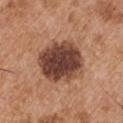Recorded during total-body skin imaging; not selected for excision or biopsy.
Longest diameter approximately 6 mm.
The lesion-visualizer software estimated a footprint of about 23 mm², a shape eccentricity near 0.55, and a symmetry-axis asymmetry near 0.1. It also reported an average lesion color of about L≈42 a*≈22 b*≈27 (CIELAB), about 18 CIELAB-L* units darker than the surrounding skin, and a normalized lesion–skin contrast near 13. The analysis additionally found a nevus-likeness score of about 75/100.
A male subject approximately 55 years of age.
This image is a 15 mm lesion crop taken from a total-body photograph.
The lesion is on the arm.
Captured under white-light illumination.